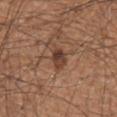No biopsy was performed on this lesion — it was imaged during a full skin examination and was not determined to be concerning.